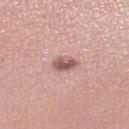Imaged during a routine full-body skin examination; the lesion was not biopsied and no histopathology is available. A female subject, aged 28–32. Longest diameter approximately 3 mm. Imaged with white-light lighting. Cropped from a total-body skin-imaging series; the visible field is about 15 mm. Automated tile analysis of the lesion measured a lesion color around L≈56 a*≈22 b*≈23 in CIELAB, roughly 14 lightness units darker than nearby skin, and a lesion-to-skin contrast of about 9 (normalized; higher = more distinct). On the leg.